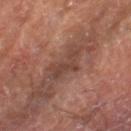Assessment:
Part of a total-body skin-imaging series; this lesion was reviewed on a skin check and was not flagged for biopsy.
Image and clinical context:
A male patient, aged around 55. About 4.5 mm across. A 15 mm close-up extracted from a 3D total-body photography capture. The lesion is located on the right lower leg.